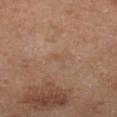{
  "biopsy_status": "not biopsied; imaged during a skin examination",
  "automated_metrics": {
    "color_variation_0_10": 0.0,
    "peripheral_color_asymmetry": 0.0,
    "nevus_likeness_0_100": 0,
    "lesion_detection_confidence_0_100": 100
  },
  "lesion_size": {
    "long_diameter_mm_approx": 1.5
  },
  "site": "left lower leg",
  "patient": {
    "sex": "female",
    "age_approx": 65
  },
  "image": {
    "source": "total-body photography crop",
    "field_of_view_mm": 15
  },
  "lighting": "cross-polarized"
}